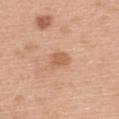notes: imaged on a skin check; not biopsied
location: the back
subject: female, roughly 60 years of age
automated metrics: a footprint of about 4 mm², an outline eccentricity of about 0.7 (0 = round, 1 = elongated), and a shape-asymmetry score of about 0.25 (0 = symmetric); internal color variation of about 1.5 on a 0–10 scale and a peripheral color-asymmetry measure near 0.5; a classifier nevus-likeness of about 25/100 and lesion-presence confidence of about 100/100
imaging modality: 15 mm crop, total-body photography
illumination: white-light
diameter: ~2.5 mm (longest diameter)